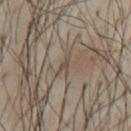Q: How was this image acquired?
A: ~15 mm tile from a whole-body skin photo
Q: Who is the patient?
A: male, approximately 40 years of age
Q: Lesion size?
A: about 1.5 mm
Q: Illumination type?
A: cross-polarized
Q: Lesion location?
A: the arm